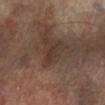<record>
  <biopsy_status>not biopsied; imaged during a skin examination</biopsy_status>
  <image>
    <source>total-body photography crop</source>
    <field_of_view_mm>15</field_of_view_mm>
  </image>
  <patient>
    <sex>female</sex>
    <age_approx>75</age_approx>
  </patient>
  <lesion_size>
    <long_diameter_mm_approx>3.0</long_diameter_mm_approx>
  </lesion_size>
  <automated_metrics>
    <area_mm2_approx>4.0</area_mm2_approx>
    <eccentricity>0.85</eccentricity>
    <cielab_L>33</cielab_L>
    <cielab_a>16</cielab_a>
    <cielab_b>22</cielab_b>
    <vs_skin_darker_L>6.0</vs_skin_darker_L>
    <vs_skin_contrast_norm>6.5</vs_skin_contrast_norm>
  </automated_metrics>
  <site>right lower leg</site>
</record>